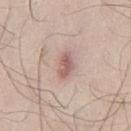- notes — catalogued during a skin exam; not biopsied
- patient — male, about 25 years old
- diameter — about 3 mm
- image — ~15 mm crop, total-body skin-cancer survey
- body site — the right thigh
- lighting — white-light illumination